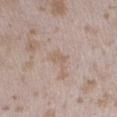  biopsy_status: not biopsied; imaged during a skin examination
  image:
    source: total-body photography crop
    field_of_view_mm: 15
  lesion_size:
    long_diameter_mm_approx: 3.0
  site: leg
  patient:
    sex: female
    age_approx: 25
  lighting: white-light